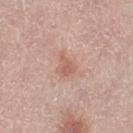Findings:
- workup: no biopsy performed (imaged during a skin exam)
- automated metrics: a mean CIELAB color near L≈60 a*≈22 b*≈27 and about 8 CIELAB-L* units darker than the surrounding skin
- illumination: white-light illumination
- imaging modality: 15 mm crop, total-body photography
- diameter: ≈3 mm
- subject: female, approximately 65 years of age
- location: the left thigh